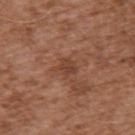Impression:
This lesion was catalogued during total-body skin photography and was not selected for biopsy.
Image and clinical context:
A male patient, aged 73–77. This is a white-light tile. From the upper back. A lesion tile, about 15 mm wide, cut from a 3D total-body photograph. Measured at roughly 3 mm in maximum diameter.